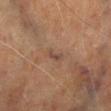The lesion was tiled from a total-body skin photograph and was not biopsied.
A male patient aged 68 to 72.
From the left lower leg.
A region of skin cropped from a whole-body photographic capture, roughly 15 mm wide.